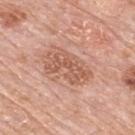The lesion was tiled from a total-body skin photograph and was not biopsied. Cropped from a total-body skin-imaging series; the visible field is about 15 mm. Automated tile analysis of the lesion measured an average lesion color of about L≈58 a*≈23 b*≈31 (CIELAB) and about 10 CIELAB-L* units darker than the surrounding skin. And it measured a nevus-likeness score of about 0/100 and a detector confidence of about 100 out of 100 that the crop contains a lesion. A male subject approximately 80 years of age. The tile uses white-light illumination. Located on the upper back.